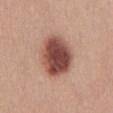workup — no biopsy performed (imaged during a skin exam)
lesion size — ~5.5 mm (longest diameter)
acquisition — ~15 mm crop, total-body skin-cancer survey
body site — the lower back
patient — male, aged around 25
image-analysis metrics — a footprint of about 19 mm², an eccentricity of roughly 0.55, and a shape-asymmetry score of about 0.15 (0 = symmetric); a classifier nevus-likeness of about 95/100 and a lesion-detection confidence of about 100/100
tile lighting — white-light